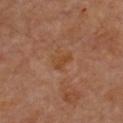Impression: Imaged during a routine full-body skin examination; the lesion was not biopsied and no histopathology is available. Image and clinical context: Longest diameter approximately 2.5 mm. Cropped from a total-body skin-imaging series; the visible field is about 15 mm. A female subject approximately 50 years of age. The total-body-photography lesion software estimated roughly 5 lightness units darker than nearby skin and a normalized lesion–skin contrast near 6.5. The analysis additionally found a border-irregularity rating of about 3/10, internal color variation of about 2 on a 0–10 scale, and radial color variation of about 0.5. From the chest. The tile uses cross-polarized illumination.